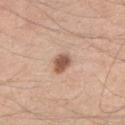follow-up = no biopsy performed (imaged during a skin exam)
imaging modality = ~15 mm tile from a whole-body skin photo
lesion size = ~3 mm (longest diameter)
subject = male, aged approximately 35
automated metrics = a lesion area of about 4.5 mm² and a shape eccentricity near 0.7; a mean CIELAB color near L≈56 a*≈21 b*≈29, roughly 15 lightness units darker than nearby skin, and a normalized border contrast of about 9.5; border irregularity of about 2 on a 0–10 scale and a peripheral color-asymmetry measure near 0.5
anatomic site = the arm
tile lighting = white-light illumination